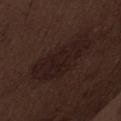workup: no biopsy performed (imaged during a skin exam) | image source: ~15 mm crop, total-body skin-cancer survey | site: the abdomen | subject: male, aged around 70.